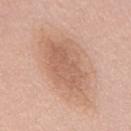<record>
<biopsy_status>not biopsied; imaged during a skin examination</biopsy_status>
<site>mid back</site>
<lesion_size>
  <long_diameter_mm_approx>8.0</long_diameter_mm_approx>
</lesion_size>
<image>
  <source>total-body photography crop</source>
  <field_of_view_mm>15</field_of_view_mm>
</image>
<patient>
  <sex>male</sex>
  <age_approx>55</age_approx>
</patient>
</record>